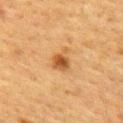Case summary:
* follow-up: no biopsy performed (imaged during a skin exam)
* image-analysis metrics: a nevus-likeness score of about 95/100 and a lesion-detection confidence of about 100/100
* size: ~3 mm (longest diameter)
* lighting: cross-polarized illumination
* image: total-body-photography crop, ~15 mm field of view
* subject: female, aged 53–57
* location: the upper back